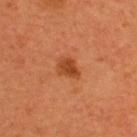The lesion was tiled from a total-body skin photograph and was not biopsied.
A female patient, aged 48–52.
Cropped from a whole-body photographic skin survey; the tile spans about 15 mm.
The lesion's longest dimension is about 3 mm.
The total-body-photography lesion software estimated an area of roughly 5 mm² and a shape eccentricity near 0.65.
Captured under cross-polarized illumination.